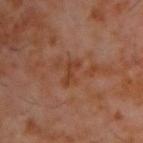Captured during whole-body skin photography for melanoma surveillance; the lesion was not biopsied.
Approximately 3 mm at its widest.
Captured under cross-polarized illumination.
The lesion is on the back.
A male patient, aged around 60.
A 15 mm close-up tile from a total-body photography series done for melanoma screening.